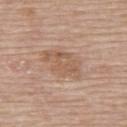Case summary:
* workup: total-body-photography surveillance lesion; no biopsy
* image: ~15 mm crop, total-body skin-cancer survey
* illumination: white-light illumination
* anatomic site: the back
* patient: female, aged 63 to 67
* TBP lesion metrics: a lesion-detection confidence of about 100/100
* diameter: ~5 mm (longest diameter)